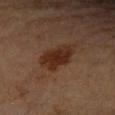{"biopsy_status": "not biopsied; imaged during a skin examination", "lesion_size": {"long_diameter_mm_approx": 5.0}, "image": {"source": "total-body photography crop", "field_of_view_mm": 15}, "patient": {"sex": "female", "age_approx": 65}, "site": "right forearm"}